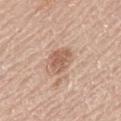Assessment: Recorded during total-body skin imaging; not selected for excision or biopsy. Background: A male subject aged 58–62. About 3 mm across. Imaged with white-light lighting. The lesion is on the mid back. A region of skin cropped from a whole-body photographic capture, roughly 15 mm wide. Automated image analysis of the tile measured a lesion area of about 6.5 mm², an eccentricity of roughly 0.35, and a symmetry-axis asymmetry near 0.2. The analysis additionally found a lesion color around L≈59 a*≈20 b*≈29 in CIELAB, about 10 CIELAB-L* units darker than the surrounding skin, and a normalized border contrast of about 7. The analysis additionally found a border-irregularity rating of about 2/10, internal color variation of about 3 on a 0–10 scale, and radial color variation of about 1.